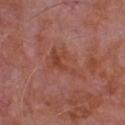Impression: No biopsy was performed on this lesion — it was imaged during a full skin examination and was not determined to be concerning. Image and clinical context: A male patient aged 63 to 67. This is a white-light tile. A region of skin cropped from a whole-body photographic capture, roughly 15 mm wide. From the chest. The lesion-visualizer software estimated a lesion color around L≈45 a*≈26 b*≈29 in CIELAB, roughly 6 lightness units darker than nearby skin, and a normalized border contrast of about 6.